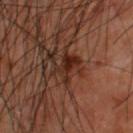The lesion was photographed on a routine skin check and not biopsied; there is no pathology result. On the back. A 15 mm close-up tile from a total-body photography series done for melanoma screening. A male patient about 60 years old. Imaged with cross-polarized lighting.